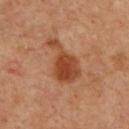{"automated_metrics": {"area_mm2_approx": 12.0, "eccentricity": 0.85}, "patient": {"sex": "male", "age_approx": 50}, "lesion_size": {"long_diameter_mm_approx": 6.0}, "lighting": "cross-polarized", "site": "chest", "image": {"source": "total-body photography crop", "field_of_view_mm": 15}}